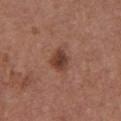Part of a total-body skin-imaging series; this lesion was reviewed on a skin check and was not flagged for biopsy. From the chest. This is a white-light tile. A female subject aged approximately 65. A 15 mm crop from a total-body photograph taken for skin-cancer surveillance.